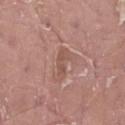| feature | finding |
|---|---|
| workup | catalogued during a skin exam; not biopsied |
| image source | ~15 mm crop, total-body skin-cancer survey |
| site | the leg |
| subject | male, in their 40s |